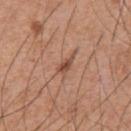About 2.5 mm across.
A 15 mm close-up extracted from a 3D total-body photography capture.
A male patient aged approximately 55.
The lesion is on the chest.
Imaged with white-light lighting.
The lesion-visualizer software estimated an eccentricity of roughly 0.85. And it measured a border-irregularity rating of about 3.5/10, a color-variation rating of about 0.5/10, and a peripheral color-asymmetry measure near 0.